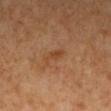- follow-up: imaged on a skin check; not biopsied
- patient: female, in their 50s
- location: the left lower leg
- lesion size: about 3 mm
- lighting: cross-polarized
- image source: total-body-photography crop, ~15 mm field of view